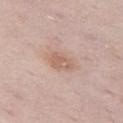Q: Was this lesion biopsied?
A: catalogued during a skin exam; not biopsied
Q: Lesion location?
A: the left thigh
Q: Illumination type?
A: white-light
Q: Who is the patient?
A: female, aged 28 to 32
Q: What did automated image analysis measure?
A: a footprint of about 5 mm², an outline eccentricity of about 0.75 (0 = round, 1 = elongated), and a shape-asymmetry score of about 0.3 (0 = symmetric); a mean CIELAB color near L≈61 a*≈19 b*≈26 and a lesion-to-skin contrast of about 5.5 (normalized; higher = more distinct); a classifier nevus-likeness of about 20/100 and lesion-presence confidence of about 100/100
Q: How large is the lesion?
A: ≈3 mm
Q: How was this image acquired?
A: ~15 mm crop, total-body skin-cancer survey